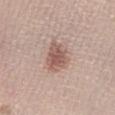Recorded during total-body skin imaging; not selected for excision or biopsy.
On the leg.
A lesion tile, about 15 mm wide, cut from a 3D total-body photograph.
Automated image analysis of the tile measured a lesion area of about 9 mm², an outline eccentricity of about 0.7 (0 = round, 1 = elongated), and a shape-asymmetry score of about 0.25 (0 = symmetric). The analysis additionally found a border-irregularity rating of about 3/10, internal color variation of about 3 on a 0–10 scale, and peripheral color asymmetry of about 1.
The tile uses white-light illumination.
The lesion's longest dimension is about 4 mm.
A female subject, in their 30s.